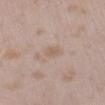Q: Is there a histopathology result?
A: catalogued during a skin exam; not biopsied
Q: Where on the body is the lesion?
A: the left lower leg
Q: What lighting was used for the tile?
A: white-light illumination
Q: What is the imaging modality?
A: ~15 mm crop, total-body skin-cancer survey
Q: Patient demographics?
A: female, aged around 25
Q: Lesion size?
A: ≈2.5 mm
Q: Automated lesion metrics?
A: an average lesion color of about L≈60 a*≈15 b*≈26 (CIELAB), roughly 6 lightness units darker than nearby skin, and a normalized lesion–skin contrast near 5; a nevus-likeness score of about 0/100